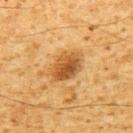Part of a total-body skin-imaging series; this lesion was reviewed on a skin check and was not flagged for biopsy. An algorithmic analysis of the crop reported a footprint of about 9.5 mm². The software also gave a mean CIELAB color near L≈45 a*≈20 b*≈38, roughly 12 lightness units darker than nearby skin, and a normalized lesion–skin contrast near 9. And it measured a peripheral color-asymmetry measure near 1.5. And it measured a nevus-likeness score of about 80/100 and a detector confidence of about 100 out of 100 that the crop contains a lesion. A male patient, in their 60s. A 15 mm close-up tile from a total-body photography series done for melanoma screening. Longest diameter approximately 4.5 mm. Imaged with cross-polarized lighting. On the upper back.